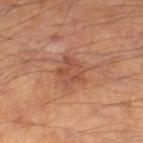workup = catalogued during a skin exam; not biopsied | anatomic site = the right lower leg | patient = male, in their mid- to late 60s | imaging modality = ~15 mm crop, total-body skin-cancer survey | lighting = cross-polarized illumination.